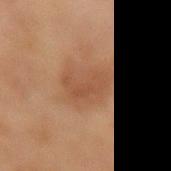{
  "biopsy_status": "not biopsied; imaged during a skin examination",
  "site": "left forearm",
  "lighting": "cross-polarized",
  "patient": {
    "sex": "female",
    "age_approx": 80
  },
  "lesion_size": {
    "long_diameter_mm_approx": 5.0
  },
  "image": {
    "source": "total-body photography crop",
    "field_of_view_mm": 15
  }
}